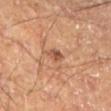Assessment:
Part of a total-body skin-imaging series; this lesion was reviewed on a skin check and was not flagged for biopsy.
Image and clinical context:
The lesion is on the left lower leg. Automated image analysis of the tile measured a footprint of about 2.5 mm², an outline eccentricity of about 0.5 (0 = round, 1 = elongated), and a shape-asymmetry score of about 0.3 (0 = symmetric). The analysis additionally found a lesion color around L≈47 a*≈21 b*≈30 in CIELAB, about 10 CIELAB-L* units darker than the surrounding skin, and a lesion-to-skin contrast of about 7.5 (normalized; higher = more distinct). The analysis additionally found a border-irregularity index near 2.5/10 and radial color variation of about 1. A male subject aged around 60. Longest diameter approximately 2 mm. This is a cross-polarized tile. A 15 mm close-up extracted from a 3D total-body photography capture.